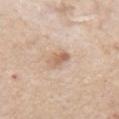Image and clinical context: Cropped from a total-body skin-imaging series; the visible field is about 15 mm. The subject is a male aged around 85 or older. From the left upper arm.